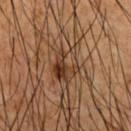| field | value |
|---|---|
| biopsy status | no biopsy performed (imaged during a skin exam) |
| image | total-body-photography crop, ~15 mm field of view |
| lesion diameter | ~4 mm (longest diameter) |
| subject | male, approximately 45 years of age |
| anatomic site | the front of the torso |